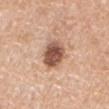Case summary:
– biopsy status · total-body-photography surveillance lesion; no biopsy
– illumination · white-light illumination
– patient · male, about 70 years old
– image source · total-body-photography crop, ~15 mm field of view
– location · the mid back
– lesion size · ~4 mm (longest diameter)